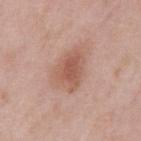Impression: Imaged during a routine full-body skin examination; the lesion was not biopsied and no histopathology is available. Clinical summary: On the mid back. A male subject roughly 55 years of age. Longest diameter approximately 5 mm. A 15 mm close-up extracted from a 3D total-body photography capture.